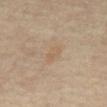follow-up = catalogued during a skin exam; not biopsied
subject = male, aged 58 to 62
illumination = cross-polarized illumination
lesion diameter = ~2.5 mm (longest diameter)
anatomic site = the abdomen
image = 15 mm crop, total-body photography